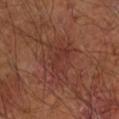follow-up = catalogued during a skin exam; not biopsied | tile lighting = cross-polarized illumination | patient = male, aged 63–67 | image source = ~15 mm crop, total-body skin-cancer survey | site = the right forearm | lesion diameter = about 5.5 mm.